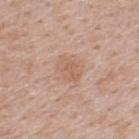Case summary:
• notes: imaged on a skin check; not biopsied
• subject: male, aged 48 to 52
• location: the back
• tile lighting: white-light
• acquisition: ~15 mm tile from a whole-body skin photo
• size: about 3 mm